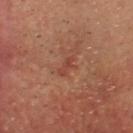<record>
<biopsy_status>not biopsied; imaged during a skin examination</biopsy_status>
<patient>
  <sex>male</sex>
  <age_approx>70</age_approx>
</patient>
<image>
  <source>total-body photography crop</source>
  <field_of_view_mm>15</field_of_view_mm>
</image>
<lighting>cross-polarized</lighting>
<site>front of the torso</site>
</record>